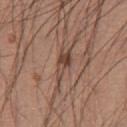Imaged during a routine full-body skin examination; the lesion was not biopsied and no histopathology is available.
The lesion is on the front of the torso.
This image is a 15 mm lesion crop taken from a total-body photograph.
A male patient, roughly 60 years of age.